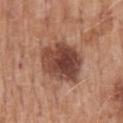Notes:
- notes: imaged on a skin check; not biopsied
- acquisition: ~15 mm crop, total-body skin-cancer survey
- tile lighting: white-light illumination
- subject: male, about 70 years old
- size: ~5.5 mm (longest diameter)
- site: the mid back